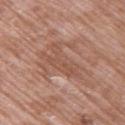<case>
  <biopsy_status>not biopsied; imaged during a skin examination</biopsy_status>
  <image>
    <source>total-body photography crop</source>
    <field_of_view_mm>15</field_of_view_mm>
  </image>
  <lighting>white-light</lighting>
  <patient>
    <sex>female</sex>
    <age_approx>70</age_approx>
  </patient>
  <lesion_size>
    <long_diameter_mm_approx>5.5</long_diameter_mm_approx>
  </lesion_size>
  <site>chest</site>
  <automated_metrics>
    <area_mm2_approx>7.5</area_mm2_approx>
    <eccentricity>0.9</eccentricity>
    <vs_skin_contrast_norm>4.5</vs_skin_contrast_norm>
    <border_irregularity_0_10>7.5</border_irregularity_0_10>
    <color_variation_0_10>2.0</color_variation_0_10>
    <peripheral_color_asymmetry>0.5</peripheral_color_asymmetry>
    <nevus_likeness_0_100>0</nevus_likeness_0_100>
  </automated_metrics>
</case>